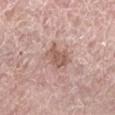Impression: Imaged during a routine full-body skin examination; the lesion was not biopsied and no histopathology is available. Clinical summary: From the left lower leg. A close-up tile cropped from a whole-body skin photograph, about 15 mm across. Measured at roughly 3.5 mm in maximum diameter. The lesion-visualizer software estimated a footprint of about 7.5 mm², an eccentricity of roughly 0.7, and a shape-asymmetry score of about 0.25 (0 = symmetric). A female patient aged 63 to 67. Imaged with white-light lighting.